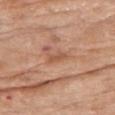Clinical impression:
The lesion was tiled from a total-body skin photograph and was not biopsied.
Image and clinical context:
The lesion is on the left upper arm. Captured under white-light illumination. The lesion-visualizer software estimated a lesion color around L≈54 a*≈23 b*≈33 in CIELAB, about 8 CIELAB-L* units darker than the surrounding skin, and a lesion-to-skin contrast of about 5.5 (normalized; higher = more distinct). The software also gave a classifier nevus-likeness of about 0/100 and a lesion-detection confidence of about 100/100. A 15 mm close-up extracted from a 3D total-body photography capture. About 3 mm across. A female patient roughly 75 years of age.